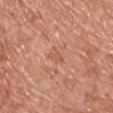The lesion was photographed on a routine skin check and not biopsied; there is no pathology result. The lesion's longest dimension is about 2.5 mm. The patient is a male about 70 years old. The lesion is located on the chest. Cropped from a total-body skin-imaging series; the visible field is about 15 mm.